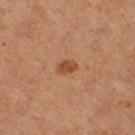Clinical summary: A close-up tile cropped from a whole-body skin photograph, about 15 mm across. A female patient aged around 65. On the right lower leg.